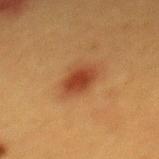biopsy status=no biopsy performed (imaged during a skin exam) | subject=female, about 40 years old | image source=~15 mm tile from a whole-body skin photo | image-analysis metrics=about 10 CIELAB-L* units darker than the surrounding skin and a normalized border contrast of about 9; a border-irregularity index near 1.5/10 and radial color variation of about 0.5 | lesion diameter=~3.5 mm (longest diameter) | anatomic site=the mid back.